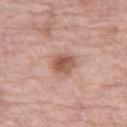Imaged with white-light lighting. A female subject, approximately 70 years of age. From the right thigh. The lesion-visualizer software estimated a footprint of about 6 mm², an eccentricity of roughly 0.55, and a shape-asymmetry score of about 0.15 (0 = symmetric). And it measured a lesion color around L≈55 a*≈23 b*≈28 in CIELAB, a lesion–skin lightness drop of about 12, and a normalized border contrast of about 8.5. It also reported border irregularity of about 1.5 on a 0–10 scale, a within-lesion color-variation index near 5/10, and radial color variation of about 2. And it measured an automated nevus-likeness rating near 85 out of 100. A 15 mm close-up tile from a total-body photography series done for melanoma screening. About 3 mm across.